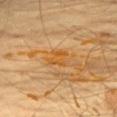{
  "biopsy_status": "not biopsied; imaged during a skin examination"
}